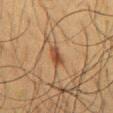The lesion was photographed on a routine skin check and not biopsied; there is no pathology result.
Longest diameter approximately 2.5 mm.
Imaged with cross-polarized lighting.
Located on the chest.
The subject is a male roughly 60 years of age.
This image is a 15 mm lesion crop taken from a total-body photograph.